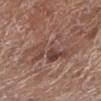Recorded during total-body skin imaging; not selected for excision or biopsy. The lesion is located on the left lower leg. About 7 mm across. Automated tile analysis of the lesion measured a lesion color around L≈46 a*≈18 b*≈24 in CIELAB. The software also gave border irregularity of about 6 on a 0–10 scale and a within-lesion color-variation index near 7.5/10. And it measured a detector confidence of about 80 out of 100 that the crop contains a lesion. A region of skin cropped from a whole-body photographic capture, roughly 15 mm wide. The subject is a female about 80 years old.